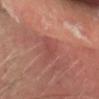notes: total-body-photography surveillance lesion; no biopsy
imaging modality: ~15 mm tile from a whole-body skin photo
lighting: cross-polarized
diameter: ~4 mm (longest diameter)
subject: male, roughly 65 years of age
body site: the head or neck
automated lesion analysis: a footprint of about 5.5 mm², a shape eccentricity near 0.85, and a shape-asymmetry score of about 0.45 (0 = symmetric); an automated nevus-likeness rating near 0 out of 100